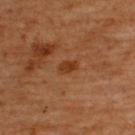Part of a total-body skin-imaging series; this lesion was reviewed on a skin check and was not flagged for biopsy.